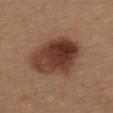No biopsy was performed on this lesion — it was imaged during a full skin examination and was not determined to be concerning. Captured under white-light illumination. A female subject in their 40s. The lesion's longest dimension is about 7.5 mm. The total-body-photography lesion software estimated a footprint of about 27 mm², a shape eccentricity near 0.75, and a symmetry-axis asymmetry near 0.2. It also reported internal color variation of about 8 on a 0–10 scale and peripheral color asymmetry of about 3. It also reported a nevus-likeness score of about 95/100 and lesion-presence confidence of about 100/100. On the upper back. A 15 mm crop from a total-body photograph taken for skin-cancer surveillance.